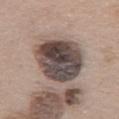- subject · female, aged around 60
- lesion diameter · about 7 mm
- anatomic site · the front of the torso
- illumination · white-light illumination
- image · ~15 mm tile from a whole-body skin photo